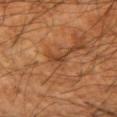Case summary:
* biopsy status · catalogued during a skin exam; not biopsied
* image-analysis metrics · a lesion color around L≈41 a*≈22 b*≈34 in CIELAB and a normalized border contrast of about 6.5; a classifier nevus-likeness of about 0/100 and a lesion-detection confidence of about 85/100
* anatomic site · the arm
* diameter · ≈2.5 mm
* image · 15 mm crop, total-body photography
* patient · male, aged approximately 45